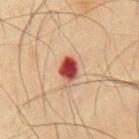This lesion was catalogued during total-body skin photography and was not selected for biopsy.